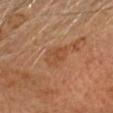Impression: No biopsy was performed on this lesion — it was imaged during a full skin examination and was not determined to be concerning. Clinical summary: Cropped from a whole-body photographic skin survey; the tile spans about 15 mm. The patient is a male in their mid- to late 60s. About 3.5 mm across. Located on the head or neck.